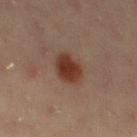workup = total-body-photography surveillance lesion; no biopsy | anatomic site = the left thigh | patient = female, aged 18–22 | image source = ~15 mm crop, total-body skin-cancer survey.